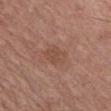Notes:
- follow-up — catalogued during a skin exam; not biopsied
- image — ~15 mm crop, total-body skin-cancer survey
- patient — male, in their mid- to late 50s
- location — the chest